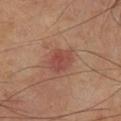follow-up = no biopsy performed (imaged during a skin exam); patient = male, approximately 65 years of age; image source = total-body-photography crop, ~15 mm field of view; tile lighting = cross-polarized illumination; body site = the right lower leg.